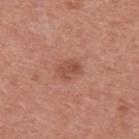The lesion was tiled from a total-body skin photograph and was not biopsied. A male patient approximately 50 years of age. The lesion is on the upper back. A lesion tile, about 15 mm wide, cut from a 3D total-body photograph. Imaged with white-light lighting.